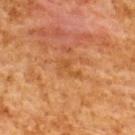Assessment:
The lesion was tiled from a total-body skin photograph and was not biopsied.
Context:
A male subject, aged 58–62. Longest diameter approximately 3 mm. A lesion tile, about 15 mm wide, cut from a 3D total-body photograph. The lesion is on the upper back.